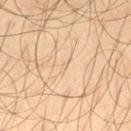Cropped from a total-body skin-imaging series; the visible field is about 15 mm. A male patient about 45 years old. Longest diameter approximately 1 mm. Located on the left thigh. This is a cross-polarized tile.